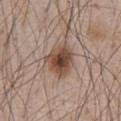The lesion was tiled from a total-body skin photograph and was not biopsied. Located on the chest. The lesion's longest dimension is about 4.5 mm. A lesion tile, about 15 mm wide, cut from a 3D total-body photograph. A male subject aged 63 to 67. The lesion-visualizer software estimated a symmetry-axis asymmetry near 0.2. The software also gave a classifier nevus-likeness of about 100/100 and a lesion-detection confidence of about 100/100. Captured under white-light illumination.